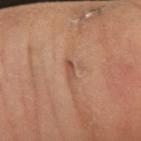Clinical impression: The lesion was tiled from a total-body skin photograph and was not biopsied. Image and clinical context: A 15 mm close-up extracted from a 3D total-body photography capture. The subject is a male approximately 40 years of age. The lesion is on the left forearm. The recorded lesion diameter is about 2.5 mm. Captured under cross-polarized illumination. An algorithmic analysis of the crop reported a lesion color around L≈50 a*≈23 b*≈30 in CIELAB, about 9 CIELAB-L* units darker than the surrounding skin, and a normalized lesion–skin contrast near 6.5. The software also gave a classifier nevus-likeness of about 0/100 and a lesion-detection confidence of about 85/100.